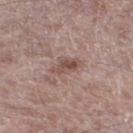biopsy status: total-body-photography surveillance lesion; no biopsy | TBP lesion metrics: an area of roughly 4.5 mm², an outline eccentricity of about 0.9 (0 = round, 1 = elongated), and a symmetry-axis asymmetry near 0.3; a border-irregularity rating of about 3.5/10, internal color variation of about 3 on a 0–10 scale, and peripheral color asymmetry of about 1 | acquisition: 15 mm crop, total-body photography | lesion size: about 3.5 mm | subject: male, aged around 70 | location: the leg.